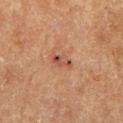Case summary:
– subject · male, in their mid-60s
– diameter · ~3 mm (longest diameter)
– image source · total-body-photography crop, ~15 mm field of view
– location · the left lower leg
– lighting · cross-polarized illumination